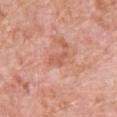Q: Is there a histopathology result?
A: no biopsy performed (imaged during a skin exam)
Q: How was this image acquired?
A: ~15 mm tile from a whole-body skin photo
Q: Who is the patient?
A: male, approximately 50 years of age
Q: Lesion location?
A: the back